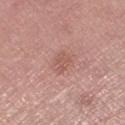Q: What are the patient's age and sex?
A: female, aged around 65
Q: Where on the body is the lesion?
A: the leg
Q: What is the imaging modality?
A: ~15 mm tile from a whole-body skin photo